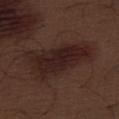{
  "lighting": "white-light",
  "automated_metrics": {
    "area_mm2_approx": 17.0,
    "eccentricity": 0.95,
    "shape_asymmetry": 0.3,
    "cielab_L": 20,
    "cielab_a": 17,
    "cielab_b": 17,
    "vs_skin_darker_L": 9.0,
    "vs_skin_contrast_norm": 11.0
  },
  "lesion_size": {
    "long_diameter_mm_approx": 7.5
  },
  "patient": {
    "sex": "male",
    "age_approx": 70
  },
  "image": {
    "source": "total-body photography crop",
    "field_of_view_mm": 15
  },
  "site": "front of the torso"
}